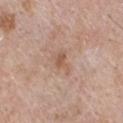Impression:
Imaged during a routine full-body skin examination; the lesion was not biopsied and no histopathology is available.
Background:
A roughly 15 mm field-of-view crop from a total-body skin photograph. Approximately 3 mm at its widest. Located on the chest. The subject is a male in their mid- to late 60s. The tile uses white-light illumination.